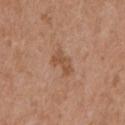Notes:
– biopsy status — no biopsy performed (imaged during a skin exam)
– acquisition — ~15 mm crop, total-body skin-cancer survey
– image-analysis metrics — an area of roughly 4.5 mm², an eccentricity of roughly 0.85, and a shape-asymmetry score of about 0.35 (0 = symmetric); a border-irregularity rating of about 4/10, internal color variation of about 1.5 on a 0–10 scale, and radial color variation of about 0.5
– body site — the left upper arm
– tile lighting — white-light illumination
– patient — male, approximately 55 years of age
– lesion diameter — ~3 mm (longest diameter)